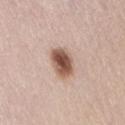Case summary:
– notes — total-body-photography surveillance lesion; no biopsy
– anatomic site — the back
– acquisition — ~15 mm tile from a whole-body skin photo
– patient — female, aged around 65
– lighting — white-light illumination